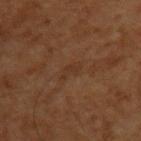• workup: total-body-photography surveillance lesion; no biopsy
• subject: male, in their 60s
• lesion size: about 3 mm
• automated metrics: a lesion color around L≈30 a*≈17 b*≈27 in CIELAB, a lesion–skin lightness drop of about 4, and a lesion-to-skin contrast of about 4.5 (normalized; higher = more distinct); border irregularity of about 4.5 on a 0–10 scale, internal color variation of about 0 on a 0–10 scale, and radial color variation of about 0
• image: ~15 mm crop, total-body skin-cancer survey
• tile lighting: cross-polarized
• anatomic site: the left upper arm